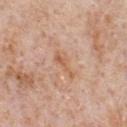biopsy status: total-body-photography surveillance lesion; no biopsy | image source: total-body-photography crop, ~15 mm field of view | automated lesion analysis: an area of roughly 4 mm², an outline eccentricity of about 0.95 (0 = round, 1 = elongated), and two-axis asymmetry of about 0.45; a lesion color around L≈60 a*≈22 b*≈34 in CIELAB, a lesion–skin lightness drop of about 8, and a normalized lesion–skin contrast near 6; a lesion-detection confidence of about 100/100 | patient: male, approximately 65 years of age | location: the chest | illumination: white-light illumination | diameter: ≈3.5 mm.